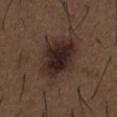Image and clinical context:
A 15 mm close-up extracted from a 3D total-body photography capture. The patient is a male about 50 years old. Automated image analysis of the tile measured an area of roughly 22 mm², a shape eccentricity near 0.7, and a symmetry-axis asymmetry near 0.2. The analysis additionally found about 11 CIELAB-L* units darker than the surrounding skin and a normalized border contrast of about 12. The software also gave an automated nevus-likeness rating near 80 out of 100 and a detector confidence of about 100 out of 100 that the crop contains a lesion. Longest diameter approximately 6.5 mm. The lesion is located on the front of the torso.